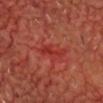workup = imaged on a skin check; not biopsied | automated metrics = border irregularity of about 6.5 on a 0–10 scale, a within-lesion color-variation index near 0.5/10, and peripheral color asymmetry of about 0; a classifier nevus-likeness of about 0/100 and a lesion-detection confidence of about 95/100 | imaging modality = ~15 mm tile from a whole-body skin photo | subject = aged 63–67 | location = the head or neck | lesion size = ~3.5 mm (longest diameter) | illumination = cross-polarized.